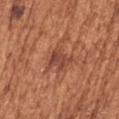The lesion was tiled from a total-body skin photograph and was not biopsied. Located on the left upper arm. A female patient, aged 73 to 77. About 3 mm across. The tile uses white-light illumination. Cropped from a whole-body photographic skin survey; the tile spans about 15 mm. An algorithmic analysis of the crop reported a mean CIELAB color near L≈46 a*≈26 b*≈30 and a normalized lesion–skin contrast near 7.5. The analysis additionally found a lesion-detection confidence of about 100/100.